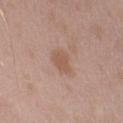{
  "site": "left upper arm",
  "patient": {
    "sex": "male",
    "age_approx": 45
  },
  "image": {
    "source": "total-body photography crop",
    "field_of_view_mm": 15
  },
  "lighting": "white-light"
}